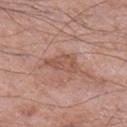biopsy_status: not biopsied; imaged during a skin examination
image:
  source: total-body photography crop
  field_of_view_mm: 15
site: right lower leg
lesion_size:
  long_diameter_mm_approx: 4.5
patient:
  sex: male
  age_approx: 60
lighting: white-light